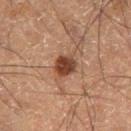illumination: cross-polarized | TBP lesion metrics: a footprint of about 5.5 mm², an outline eccentricity of about 0.45 (0 = round, 1 = elongated), and two-axis asymmetry of about 0.2; an average lesion color of about L≈38 a*≈22 b*≈28 (CIELAB) and about 14 CIELAB-L* units darker than the surrounding skin; border irregularity of about 1.5 on a 0–10 scale, a within-lesion color-variation index near 3.5/10, and a peripheral color-asymmetry measure near 1; an automated nevus-likeness rating near 95 out of 100 and a lesion-detection confidence of about 100/100 | subject: male, aged approximately 60 | location: the left thigh | image: 15 mm crop, total-body photography.